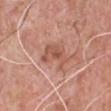biopsy status: total-body-photography surveillance lesion; no biopsy | anatomic site: the chest | acquisition: ~15 mm crop, total-body skin-cancer survey | lighting: white-light illumination | subject: male, aged 58 to 62 | lesion diameter: ~4 mm (longest diameter) | automated metrics: a lesion area of about 8 mm², a shape eccentricity near 0.65, and a symmetry-axis asymmetry near 0.55; a lesion color around L≈55 a*≈24 b*≈29 in CIELAB, a lesion–skin lightness drop of about 9, and a lesion-to-skin contrast of about 6 (normalized; higher = more distinct); a nevus-likeness score of about 0/100 and lesion-presence confidence of about 100/100.